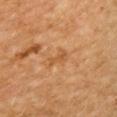Recorded during total-body skin imaging; not selected for excision or biopsy.
A 15 mm close-up tile from a total-body photography series done for melanoma screening.
The lesion is located on the upper back.
Imaged with cross-polarized lighting.
A female subject, aged 53–57.